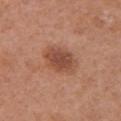Case summary:
• biopsy status — total-body-photography surveillance lesion; no biopsy
• image source — 15 mm crop, total-body photography
• location — the front of the torso
• lighting — white-light illumination
• diameter — ≈4.5 mm
• subject — female, aged 48–52
• TBP lesion metrics — a lesion color around L≈49 a*≈24 b*≈30 in CIELAB, a lesion–skin lightness drop of about 10, and a normalized lesion–skin contrast near 7.5; an automated nevus-likeness rating near 90 out of 100 and lesion-presence confidence of about 100/100